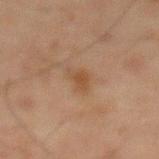biopsy status = total-body-photography surveillance lesion; no biopsy | anatomic site = the front of the torso | patient = male, aged 73 to 77 | acquisition = 15 mm crop, total-body photography.